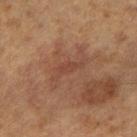Notes:
– follow-up: no biopsy performed (imaged during a skin exam)